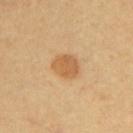Impression: The lesion was tiled from a total-body skin photograph and was not biopsied. Clinical summary: Cropped from a total-body skin-imaging series; the visible field is about 15 mm. From the upper back. This is a cross-polarized tile. Measured at roughly 3 mm in maximum diameter. A patient aged around 60.